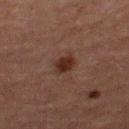Part of a total-body skin-imaging series; this lesion was reviewed on a skin check and was not flagged for biopsy. Located on the left thigh. Approximately 2.5 mm at its widest. Imaged with cross-polarized lighting. This image is a 15 mm lesion crop taken from a total-body photograph. A female subject, approximately 60 years of age. The total-body-photography lesion software estimated a lesion color around L≈23 a*≈17 b*≈21 in CIELAB, a lesion–skin lightness drop of about 8, and a lesion-to-skin contrast of about 9.5 (normalized; higher = more distinct). The analysis additionally found a classifier nevus-likeness of about 90/100 and lesion-presence confidence of about 100/100.